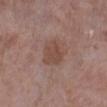Part of a total-body skin-imaging series; this lesion was reviewed on a skin check and was not flagged for biopsy. A 15 mm close-up extracted from a 3D total-body photography capture. The patient is a male about 70 years old. Measured at roughly 3 mm in maximum diameter. Captured under white-light illumination. The lesion is located on the left lower leg.